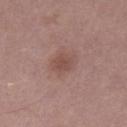Part of a total-body skin-imaging series; this lesion was reviewed on a skin check and was not flagged for biopsy. This is a white-light tile. A male patient, in their 70s. A 15 mm crop from a total-body photograph taken for skin-cancer surveillance. The lesion's longest dimension is about 3 mm. The lesion is located on the right thigh.